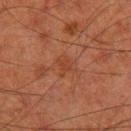Q: Was a biopsy performed?
A: imaged on a skin check; not biopsied
Q: What did automated image analysis measure?
A: an area of roughly 4 mm², a shape eccentricity near 0.5, and a shape-asymmetry score of about 0.4 (0 = symmetric); a border-irregularity rating of about 4/10, internal color variation of about 2 on a 0–10 scale, and radial color variation of about 0.5; an automated nevus-likeness rating near 0 out of 100 and a detector confidence of about 100 out of 100 that the crop contains a lesion
Q: Who is the patient?
A: male, approximately 80 years of age
Q: Lesion location?
A: the right thigh
Q: What lighting was used for the tile?
A: cross-polarized
Q: What is the imaging modality?
A: ~15 mm tile from a whole-body skin photo
Q: What is the lesion's diameter?
A: about 2.5 mm